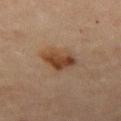Assessment:
Imaged during a routine full-body skin examination; the lesion was not biopsied and no histopathology is available.
Context:
A roughly 15 mm field-of-view crop from a total-body skin photograph. The lesion is located on the leg. A female subject aged approximately 70.